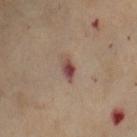The lesion was tiled from a total-body skin photograph and was not biopsied. This image is a 15 mm lesion crop taken from a total-body photograph. Automated image analysis of the tile measured a lesion area of about 3.5 mm², an outline eccentricity of about 0.85 (0 = round, 1 = elongated), and a shape-asymmetry score of about 0.2 (0 = symmetric). It also reported a lesion color around L≈47 a*≈21 b*≈22 in CIELAB, roughly 12 lightness units darker than nearby skin, and a lesion-to-skin contrast of about 9.5 (normalized; higher = more distinct). Captured under cross-polarized illumination. Longest diameter approximately 3 mm. The patient is a female roughly 55 years of age. The lesion is located on the arm.